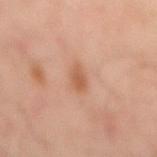<case>
<biopsy_status>not biopsied; imaged during a skin examination</biopsy_status>
<site>mid back</site>
<image>
  <source>total-body photography crop</source>
  <field_of_view_mm>15</field_of_view_mm>
</image>
<lesion_size>
  <long_diameter_mm_approx>3.0</long_diameter_mm_approx>
</lesion_size>
<patient>
  <sex>male</sex>
  <age_approx>50</age_approx>
</patient>
</case>